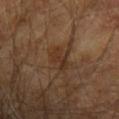Q: Was a biopsy performed?
A: imaged on a skin check; not biopsied
Q: How large is the lesion?
A: ≈3.5 mm
Q: What is the imaging modality?
A: 15 mm crop, total-body photography
Q: Who is the patient?
A: male, aged 68–72
Q: How was the tile lit?
A: cross-polarized
Q: Lesion location?
A: the left forearm
Q: What did automated image analysis measure?
A: an area of roughly 4.5 mm², an eccentricity of roughly 0.85, and a symmetry-axis asymmetry near 0.35; a within-lesion color-variation index near 2/10 and peripheral color asymmetry of about 0.5; a classifier nevus-likeness of about 0/100